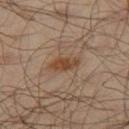Impression:
This lesion was catalogued during total-body skin photography and was not selected for biopsy.
Background:
On the right thigh. The patient is a male aged approximately 65. The lesion's longest dimension is about 4 mm. The lesion-visualizer software estimated a lesion area of about 5.5 mm², a shape eccentricity near 0.9, and a symmetry-axis asymmetry near 0.25. And it measured roughly 9 lightness units darker than nearby skin. The software also gave a classifier nevus-likeness of about 75/100 and a lesion-detection confidence of about 100/100. Imaged with cross-polarized lighting. This image is a 15 mm lesion crop taken from a total-body photograph.